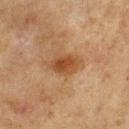workup = no biopsy performed (imaged during a skin exam).